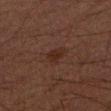{
  "biopsy_status": "not biopsied; imaged during a skin examination",
  "patient": {
    "sex": "male",
    "age_approx": 50
  },
  "image": {
    "source": "total-body photography crop",
    "field_of_view_mm": 15
  },
  "lesion_size": {
    "long_diameter_mm_approx": 2.5
  },
  "lighting": "cross-polarized",
  "automated_metrics": {
    "border_irregularity_0_10": 3.0,
    "color_variation_0_10": 2.0,
    "peripheral_color_asymmetry": 0.5
  },
  "site": "right upper arm"
}